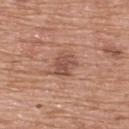follow-up=total-body-photography surveillance lesion; no biopsy
acquisition=15 mm crop, total-body photography
location=the upper back
TBP lesion metrics=a border-irregularity index near 2.5/10 and a within-lesion color-variation index near 3/10
illumination=white-light illumination
patient=male, aged 58–62